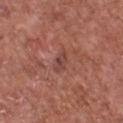Case summary:
• biopsy status: total-body-photography surveillance lesion; no biopsy
• acquisition: 15 mm crop, total-body photography
• automated lesion analysis: an average lesion color of about L≈43 a*≈23 b*≈25 (CIELAB), a lesion–skin lightness drop of about 8, and a normalized border contrast of about 6.5; a border-irregularity index near 3/10, a color-variation rating of about 0/10, and peripheral color asymmetry of about 0; a classifier nevus-likeness of about 0/100 and lesion-presence confidence of about 100/100
• tile lighting: white-light illumination
• lesion diameter: about 2.5 mm
• body site: the front of the torso
• patient: male, aged approximately 75